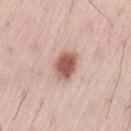Clinical impression: Recorded during total-body skin imaging; not selected for excision or biopsy. Clinical summary: On the left thigh. Cropped from a total-body skin-imaging series; the visible field is about 15 mm. This is a white-light tile. An algorithmic analysis of the crop reported an area of roughly 8.5 mm², an eccentricity of roughly 0.7, and a shape-asymmetry score of about 0.15 (0 = symmetric). The software also gave a lesion-to-skin contrast of about 9.5 (normalized; higher = more distinct). A male patient in their mid-50s.